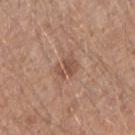| feature | finding |
|---|---|
| notes | imaged on a skin check; not biopsied |
| acquisition | 15 mm crop, total-body photography |
| lighting | white-light illumination |
| patient | male, roughly 40 years of age |
| lesion diameter | about 3 mm |
| automated metrics | a mean CIELAB color near L≈51 a*≈20 b*≈28, roughly 9 lightness units darker than nearby skin, and a normalized lesion–skin contrast near 6.5; a border-irregularity rating of about 5/10, a color-variation rating of about 1.5/10, and a peripheral color-asymmetry measure near 0.5; an automated nevus-likeness rating near 5 out of 100 |
| site | the right forearm |